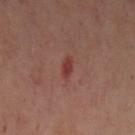The lesion was photographed on a routine skin check and not biopsied; there is no pathology result. A roughly 15 mm field-of-view crop from a total-body skin photograph. The lesion is located on the left upper arm. Automated image analysis of the tile measured an eccentricity of roughly 0.9 and two-axis asymmetry of about 0.3. And it measured a detector confidence of about 100 out of 100 that the crop contains a lesion. About 2.5 mm across. The patient is a female aged 38–42.